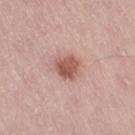– notes: catalogued during a skin exam; not biopsied
– patient: female, aged 38 to 42
– acquisition: ~15 mm tile from a whole-body skin photo
– anatomic site: the right thigh